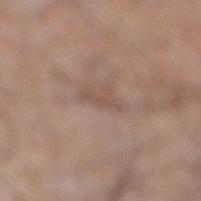tile lighting = white-light illumination; subject = male, aged 53–57; acquisition = ~15 mm tile from a whole-body skin photo; lesion size = ~3 mm (longest diameter); site = the right lower leg.